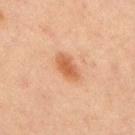Impression: The lesion was tiled from a total-body skin photograph and was not biopsied. Clinical summary: Automated tile analysis of the lesion measured an area of roughly 5.5 mm², an eccentricity of roughly 0.85, and a symmetry-axis asymmetry near 0.2. It also reported a lesion–skin lightness drop of about 9. And it measured border irregularity of about 2.5 on a 0–10 scale, internal color variation of about 2 on a 0–10 scale, and a peripheral color-asymmetry measure near 0.5. The software also gave a nevus-likeness score of about 95/100. Imaged with cross-polarized lighting. The lesion is located on the chest. This image is a 15 mm lesion crop taken from a total-body photograph. A male subject in their mid-60s.